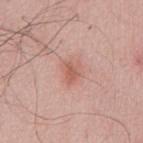This lesion was catalogued during total-body skin photography and was not selected for biopsy.
A close-up tile cropped from a whole-body skin photograph, about 15 mm across.
Imaged with white-light lighting.
The lesion is located on the chest.
A male patient, aged 58 to 62.
About 2.5 mm across.